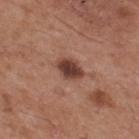<record>
  <biopsy_status>not biopsied; imaged during a skin examination</biopsy_status>
  <image>
    <source>total-body photography crop</source>
    <field_of_view_mm>15</field_of_view_mm>
  </image>
  <site>back</site>
  <lighting>white-light</lighting>
  <patient>
    <sex>male</sex>
    <age_approx>55</age_approx>
  </patient>
</record>